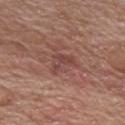biopsy_status: not biopsied; imaged during a skin examination
patient:
  sex: male
  age_approx: 70
image:
  source: total-body photography crop
  field_of_view_mm: 15
site: chest